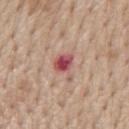Case summary:
* workup — imaged on a skin check; not biopsied
* patient — male, aged 68–72
* image source — ~15 mm tile from a whole-body skin photo
* automated metrics — a lesion area of about 5.5 mm², an eccentricity of roughly 0.7, and a shape-asymmetry score of about 0.45 (0 = symmetric)
* anatomic site — the mid back